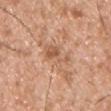The lesion was tiled from a total-body skin photograph and was not biopsied. The lesion is on the chest. About 4.5 mm across. A male subject roughly 30 years of age. This image is a 15 mm lesion crop taken from a total-body photograph.